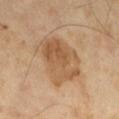<record>
  <biopsy_status>not biopsied; imaged during a skin examination</biopsy_status>
  <patient>
    <sex>male</sex>
    <age_approx>65</age_approx>
  </patient>
  <lighting>cross-polarized</lighting>
  <image>
    <source>total-body photography crop</source>
    <field_of_view_mm>15</field_of_view_mm>
  </image>
  <lesion_size>
    <long_diameter_mm_approx>6.0</long_diameter_mm_approx>
  </lesion_size>
  <automated_metrics>
    <cielab_L>54</cielab_L>
    <cielab_a>18</cielab_a>
    <cielab_b>34</cielab_b>
    <vs_skin_darker_L>9.0</vs_skin_darker_L>
    <vs_skin_contrast_norm>7.0</vs_skin_contrast_norm>
    <nevus_likeness_0_100>40</nevus_likeness_0_100>
  </automated_metrics>
</record>